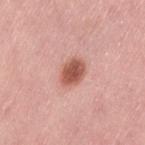<case>
<biopsy_status>not biopsied; imaged during a skin examination</biopsy_status>
<lighting>white-light</lighting>
<patient>
  <sex>female</sex>
  <age_approx>50</age_approx>
</patient>
<lesion_size>
  <long_diameter_mm_approx>3.5</long_diameter_mm_approx>
</lesion_size>
<image>
  <source>total-body photography crop</source>
  <field_of_view_mm>15</field_of_view_mm>
</image>
<automated_metrics>
  <eccentricity>0.65</eccentricity>
  <shape_asymmetry>0.15</shape_asymmetry>
  <border_irregularity_0_10>1.5</border_irregularity_0_10>
  <peripheral_color_asymmetry>1.0</peripheral_color_asymmetry>
  <nevus_likeness_0_100>100</nevus_likeness_0_100>
  <lesion_detection_confidence_0_100>100</lesion_detection_confidence_0_100>
</automated_metrics>
<site>left thigh</site>
</case>